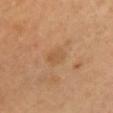Automated image analysis of the tile measured an automated nevus-likeness rating near 0 out of 100 and lesion-presence confidence of about 100/100.
Approximately 2.5 mm at its widest.
A lesion tile, about 15 mm wide, cut from a 3D total-body photograph.
Captured under cross-polarized illumination.
A female patient aged 33 to 37.
The lesion is located on the chest.